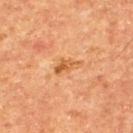<lesion>
  <biopsy_status>not biopsied; imaged during a skin examination</biopsy_status>
  <site>upper back</site>
  <lesion_size>
    <long_diameter_mm_approx>3.5</long_diameter_mm_approx>
  </lesion_size>
  <image>
    <source>total-body photography crop</source>
    <field_of_view_mm>15</field_of_view_mm>
  </image>
  <patient>
    <sex>male</sex>
    <age_approx>65</age_approx>
  </patient>
</lesion>